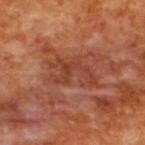The subject is a male in their mid-60s. Imaged with cross-polarized lighting. This image is a 15 mm lesion crop taken from a total-body photograph.